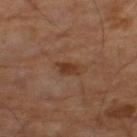Assessment: Imaged during a routine full-body skin examination; the lesion was not biopsied and no histopathology is available. Image and clinical context: The subject is a male aged 63–67. This is a cross-polarized tile. Measured at roughly 2.5 mm in maximum diameter. A 15 mm close-up tile from a total-body photography series done for melanoma screening.